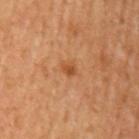follow-up = catalogued during a skin exam; not biopsied | tile lighting = cross-polarized | anatomic site = the left upper arm | subject = male, aged approximately 60 | acquisition = ~15 mm tile from a whole-body skin photo | diameter = ~1.5 mm (longest diameter).